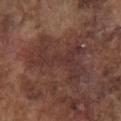  biopsy_status: not biopsied; imaged during a skin examination
  patient:
    sex: male
    age_approx: 75
  lighting: white-light
  image:
    source: total-body photography crop
    field_of_view_mm: 15
  automated_metrics:
    vs_skin_contrast_norm: 6.5
    color_variation_0_10: 3.0
    nevus_likeness_0_100: 0
  site: chest
  lesion_size:
    long_diameter_mm_approx: 7.5